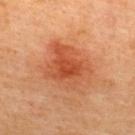This lesion was catalogued during total-body skin photography and was not selected for biopsy.
The tile uses cross-polarized illumination.
A roughly 15 mm field-of-view crop from a total-body skin photograph.
The lesion's longest dimension is about 6 mm.
A male patient about 45 years old.
The lesion is located on the upper back.